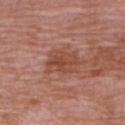The lesion was photographed on a routine skin check and not biopsied; there is no pathology result.
This image is a 15 mm lesion crop taken from a total-body photograph.
Located on the right upper arm.
Captured under white-light illumination.
The patient is a female aged 68 to 72.
Approximately 4.5 mm at its widest.